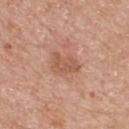The lesion was photographed on a routine skin check and not biopsied; there is no pathology result. Approximately 3.5 mm at its widest. The tile uses white-light illumination. From the upper back. A 15 mm close-up tile from a total-body photography series done for melanoma screening. The total-body-photography lesion software estimated a lesion color around L≈56 a*≈23 b*≈31 in CIELAB, roughly 8 lightness units darker than nearby skin, and a normalized lesion–skin contrast near 6. It also reported border irregularity of about 3 on a 0–10 scale, a color-variation rating of about 2.5/10, and peripheral color asymmetry of about 1. The software also gave a classifier nevus-likeness of about 0/100 and lesion-presence confidence of about 100/100. The subject is a male aged approximately 60.